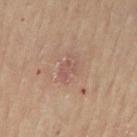follow-up — total-body-photography surveillance lesion; no biopsy
site — the left thigh
lesion size — ≈3 mm
subject — female, aged 58–62
tile lighting — cross-polarized
automated metrics — an outline eccentricity of about 0.8 (0 = round, 1 = elongated) and a symmetry-axis asymmetry near 0.35; a classifier nevus-likeness of about 0/100 and lesion-presence confidence of about 100/100
image — ~15 mm crop, total-body skin-cancer survey